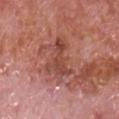| field | value |
|---|---|
| workup | catalogued during a skin exam; not biopsied |
| acquisition | 15 mm crop, total-body photography |
| lesion size | ≈5 mm |
| lighting | white-light |
| anatomic site | the chest |
| subject | male, aged 63–67 |
| image-analysis metrics | an area of roughly 8 mm² and an outline eccentricity of about 0.85 (0 = round, 1 = elongated); a mean CIELAB color near L≈46 a*≈27 b*≈28, about 9 CIELAB-L* units darker than the surrounding skin, and a lesion-to-skin contrast of about 7 (normalized; higher = more distinct) |